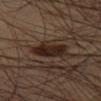Background: From the left thigh. Imaged with cross-polarized lighting. A male subject, aged around 45. A 15 mm crop from a total-body photograph taken for skin-cancer surveillance. The lesion's longest dimension is about 4 mm.